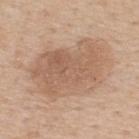Assessment: Captured during whole-body skin photography for melanoma surveillance; the lesion was not biopsied. Background: A male subject aged around 60. Imaged with white-light lighting. Located on the upper back. A roughly 15 mm field-of-view crop from a total-body skin photograph.